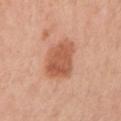Assessment: No biopsy was performed on this lesion — it was imaged during a full skin examination and was not determined to be concerning. Background: The recorded lesion diameter is about 4 mm. A 15 mm close-up tile from a total-body photography series done for melanoma screening. Captured under white-light illumination. Located on the arm. The subject is a female in their mid-50s.